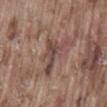follow-up = no biopsy performed (imaged during a skin exam) | TBP lesion metrics = a lesion area of about 9 mm² and an eccentricity of roughly 0.65; a lesion color around L≈45 a*≈18 b*≈22 in CIELAB, about 9 CIELAB-L* units darker than the surrounding skin, and a normalized border contrast of about 7.5; border irregularity of about 8.5 on a 0–10 scale, a within-lesion color-variation index near 3/10, and radial color variation of about 1; a nevus-likeness score of about 0/100 and lesion-presence confidence of about 50/100 | size = about 5 mm | patient = male, in their mid-70s | lighting = white-light | anatomic site = the lower back | acquisition = total-body-photography crop, ~15 mm field of view.